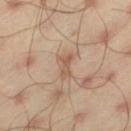Part of a total-body skin-imaging series; this lesion was reviewed on a skin check and was not flagged for biopsy. A male patient, approximately 45 years of age. A region of skin cropped from a whole-body photographic capture, roughly 15 mm wide. Longest diameter approximately 3 mm. Imaged with cross-polarized lighting. Located on the left thigh.